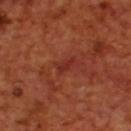This lesion was catalogued during total-body skin photography and was not selected for biopsy.
A male patient approximately 70 years of age.
The recorded lesion diameter is about 2.5 mm.
A close-up tile cropped from a whole-body skin photograph, about 15 mm across.
From the upper back.
Captured under cross-polarized illumination.